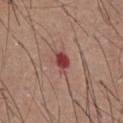The lesion was tiled from a total-body skin photograph and was not biopsied.
The lesion is on the chest.
The tile uses white-light illumination.
Cropped from a total-body skin-imaging series; the visible field is about 15 mm.
A male patient in their mid- to late 50s.
Approximately 3 mm at its widest.